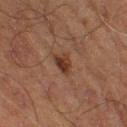{"biopsy_status": "not biopsied; imaged during a skin examination", "patient": {"sex": "male", "age_approx": 70}, "site": "right thigh", "image": {"source": "total-body photography crop", "field_of_view_mm": 15}, "lighting": "cross-polarized"}